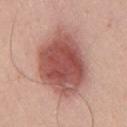The total-body-photography lesion software estimated a shape eccentricity near 0.7 and a shape-asymmetry score of about 0.15 (0 = symmetric). The analysis additionally found a lesion–skin lightness drop of about 16 and a normalized lesion–skin contrast near 10.5. The software also gave a border-irregularity index near 2/10, internal color variation of about 5 on a 0–10 scale, and peripheral color asymmetry of about 1. A 15 mm crop from a total-body photograph taken for skin-cancer surveillance. A male subject roughly 55 years of age. The tile uses white-light illumination. Approximately 8.5 mm at its widest. On the chest.